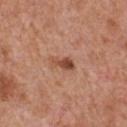subject=male, aged 63–67; size=≈3.5 mm; site=the chest; acquisition=~15 mm tile from a whole-body skin photo.